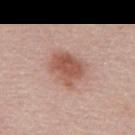No biopsy was performed on this lesion — it was imaged during a full skin examination and was not determined to be concerning. This is a white-light tile. The lesion is located on the back. Automated tile analysis of the lesion measured an area of roughly 11 mm² and an outline eccentricity of about 0.75 (0 = round, 1 = elongated). The subject is a male aged 58–62. About 4.5 mm across. A 15 mm crop from a total-body photograph taken for skin-cancer surveillance.